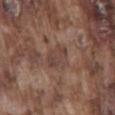Assessment:
Recorded during total-body skin imaging; not selected for excision or biopsy.
Image and clinical context:
A male subject, in their mid-70s. This is a white-light tile. A 15 mm close-up extracted from a 3D total-body photography capture. From the mid back.